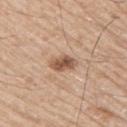Recorded during total-body skin imaging; not selected for excision or biopsy.
On the right upper arm.
A male patient aged around 75.
A close-up tile cropped from a whole-body skin photograph, about 15 mm across.